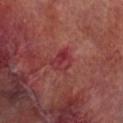Recorded during total-body skin imaging; not selected for excision or biopsy. A close-up tile cropped from a whole-body skin photograph, about 15 mm across. Captured under cross-polarized illumination. The patient is a male aged 68–72. On the right lower leg. About 2.5 mm across.